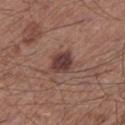notes: total-body-photography surveillance lesion; no biopsy
subject: male, aged approximately 60
lighting: white-light
diameter: ≈3 mm
automated lesion analysis: border irregularity of about 1.5 on a 0–10 scale and a within-lesion color-variation index near 4/10
body site: the leg
acquisition: total-body-photography crop, ~15 mm field of view